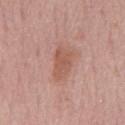Part of a total-body skin-imaging series; this lesion was reviewed on a skin check and was not flagged for biopsy. The lesion is on the chest. Cropped from a total-body skin-imaging series; the visible field is about 15 mm. A male subject, aged approximately 75.